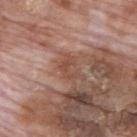This lesion was catalogued during total-body skin photography and was not selected for biopsy. Located on the upper back. The total-body-photography lesion software estimated an average lesion color of about L≈48 a*≈22 b*≈27 (CIELAB), about 7 CIELAB-L* units darker than the surrounding skin, and a normalized lesion–skin contrast near 5.5. The software also gave a border-irregularity rating of about 6.5/10, a within-lesion color-variation index near 0/10, and a peripheral color-asymmetry measure near 0. Cropped from a total-body skin-imaging series; the visible field is about 15 mm. A male subject in their 70s.